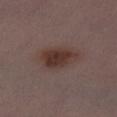- workup · catalogued during a skin exam; not biopsied
- subject · female, approximately 30 years of age
- image source · total-body-photography crop, ~15 mm field of view
- TBP lesion metrics · a border-irregularity index near 2/10, a within-lesion color-variation index near 3.5/10, and a peripheral color-asymmetry measure near 1; a detector confidence of about 100 out of 100 that the crop contains a lesion
- tile lighting · white-light illumination
- location · the right thigh